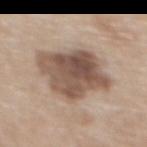follow-up: total-body-photography surveillance lesion; no biopsy | illumination: white-light illumination | patient: female, in their 60s | acquisition: ~15 mm tile from a whole-body skin photo | body site: the upper back | automated metrics: a border-irregularity index near 3/10, internal color variation of about 6.5 on a 0–10 scale, and a peripheral color-asymmetry measure near 2.5 | diameter: about 7 mm.